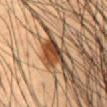image: total-body-photography crop, ~15 mm field of view | subject: male, aged around 50 | anatomic site: the abdomen | lesion size: ≈5 mm | automated lesion analysis: a footprint of about 8.5 mm² and an outline eccentricity of about 0.85 (0 = round, 1 = elongated); an average lesion color of about L≈34 a*≈17 b*≈27 (CIELAB) and a normalized border contrast of about 12; a nevus-likeness score of about 100/100 and a detector confidence of about 100 out of 100 that the crop contains a lesion.